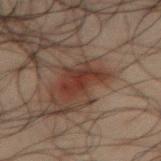Assessment:
Part of a total-body skin-imaging series; this lesion was reviewed on a skin check and was not flagged for biopsy.
Image and clinical context:
Longest diameter approximately 4 mm. Located on the abdomen. A lesion tile, about 15 mm wide, cut from a 3D total-body photograph. The patient is a male aged around 50.